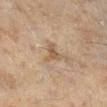acquisition: ~15 mm crop, total-body skin-cancer survey; location: the right lower leg; subject: female, aged 58–62.